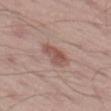Impression: No biopsy was performed on this lesion — it was imaged during a full skin examination and was not determined to be concerning. Image and clinical context: The tile uses white-light illumination. The subject is a male approximately 55 years of age. A 15 mm close-up extracted from a 3D total-body photography capture. The lesion is on the leg. The lesion-visualizer software estimated a mean CIELAB color near L≈53 a*≈21 b*≈23 and roughly 10 lightness units darker than nearby skin. The software also gave a border-irregularity rating of about 2.5/10, internal color variation of about 3.5 on a 0–10 scale, and peripheral color asymmetry of about 1. And it measured an automated nevus-likeness rating near 65 out of 100 and a detector confidence of about 100 out of 100 that the crop contains a lesion. Measured at roughly 4 mm in maximum diameter.